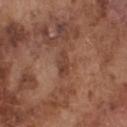notes = catalogued during a skin exam; not biopsied.